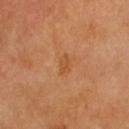Q: Was this lesion biopsied?
A: total-body-photography surveillance lesion; no biopsy
Q: Where on the body is the lesion?
A: the head or neck
Q: What kind of image is this?
A: total-body-photography crop, ~15 mm field of view
Q: What are the patient's age and sex?
A: male, in their mid-60s